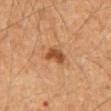The lesion was tiled from a total-body skin photograph and was not biopsied. Located on the mid back. A male subject, approximately 60 years of age. A 15 mm close-up tile from a total-body photography series done for melanoma screening. The lesion's longest dimension is about 2.5 mm.